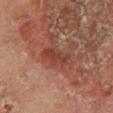{
  "biopsy_status": "not biopsied; imaged during a skin examination",
  "lighting": "cross-polarized",
  "image": {
    "source": "total-body photography crop",
    "field_of_view_mm": 15
  },
  "patient": {
    "sex": "female",
    "age_approx": 50
  },
  "lesion_size": {
    "long_diameter_mm_approx": 4.0
  },
  "site": "left lower leg"
}